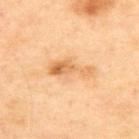The lesion was tiled from a total-body skin photograph and was not biopsied. Imaged with cross-polarized lighting. A female subject in their 40s. A 15 mm close-up tile from a total-body photography series done for melanoma screening. Approximately 5 mm at its widest. The total-body-photography lesion software estimated a footprint of about 8 mm², a shape eccentricity near 0.9, and a symmetry-axis asymmetry near 0.4. It also reported a border-irregularity index near 5/10 and a within-lesion color-variation index near 5/10. The analysis additionally found a classifier nevus-likeness of about 25/100 and a lesion-detection confidence of about 100/100. Located on the upper back.